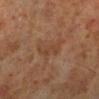Impression: No biopsy was performed on this lesion — it was imaged during a full skin examination and was not determined to be concerning. Clinical summary: A 15 mm close-up tile from a total-body photography series done for melanoma screening. Approximately 3.5 mm at its widest. From the leg. A male patient, about 60 years old. The lesion-visualizer software estimated a shape eccentricity near 0.9.